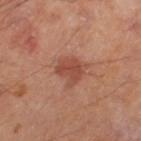Q: Was this lesion biopsied?
A: total-body-photography surveillance lesion; no biopsy
Q: What kind of image is this?
A: ~15 mm crop, total-body skin-cancer survey
Q: Where on the body is the lesion?
A: the left thigh
Q: What is the lesion's diameter?
A: ≈3 mm
Q: Illumination type?
A: cross-polarized illumination
Q: What are the patient's age and sex?
A: male, in their mid- to late 60s